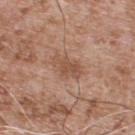Part of a total-body skin-imaging series; this lesion was reviewed on a skin check and was not flagged for biopsy.
The subject is a male about 55 years old.
Measured at roughly 3.5 mm in maximum diameter.
On the back.
A region of skin cropped from a whole-body photographic capture, roughly 15 mm wide.